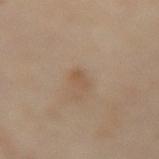Part of a total-body skin-imaging series; this lesion was reviewed on a skin check and was not flagged for biopsy.